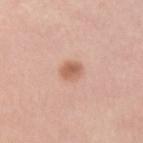– notes: no biopsy performed (imaged during a skin exam)
– body site: the arm
– patient: female, in their mid-30s
– imaging modality: 15 mm crop, total-body photography